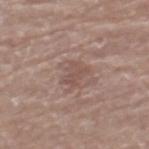Imaged during a routine full-body skin examination; the lesion was not biopsied and no histopathology is available. A male subject, roughly 80 years of age. An algorithmic analysis of the crop reported an average lesion color of about L≈51 a*≈18 b*≈22 (CIELAB), a lesion–skin lightness drop of about 7, and a normalized border contrast of about 5. It also reported border irregularity of about 5 on a 0–10 scale, a color-variation rating of about 1.5/10, and peripheral color asymmetry of about 0.5. A region of skin cropped from a whole-body photographic capture, roughly 15 mm wide. From the left thigh. Approximately 3 mm at its widest.